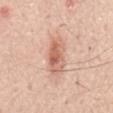Notes:
* workup: catalogued during a skin exam; not biopsied
* illumination: white-light illumination
* imaging modality: total-body-photography crop, ~15 mm field of view
* lesion size: about 5 mm
* patient: male, roughly 70 years of age
* location: the mid back